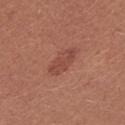workup: catalogued during a skin exam; not biopsied | body site: the left upper arm | lesion size: ~4.5 mm (longest diameter) | imaging modality: total-body-photography crop, ~15 mm field of view | lighting: white-light | subject: female, approximately 25 years of age.